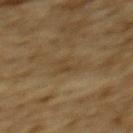{"biopsy_status": "not biopsied; imaged during a skin examination", "automated_metrics": {"color_variation_0_10": 0.0, "peripheral_color_asymmetry": 0.0, "nevus_likeness_0_100": 0}, "site": "upper back", "lesion_size": {"long_diameter_mm_approx": 3.0}, "patient": {"sex": "male", "age_approx": "85+"}, "image": {"source": "total-body photography crop", "field_of_view_mm": 15}}